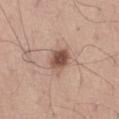No biopsy was performed on this lesion — it was imaged during a full skin examination and was not determined to be concerning.
Automated image analysis of the tile measured a normalized border contrast of about 10. It also reported internal color variation of about 4 on a 0–10 scale and a peripheral color-asymmetry measure near 1.5.
A roughly 15 mm field-of-view crop from a total-body skin photograph.
Measured at roughly 3 mm in maximum diameter.
On the leg.
This is a white-light tile.
A male patient in their mid- to late 50s.